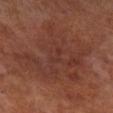The lesion was tiled from a total-body skin photograph and was not biopsied.
This is a cross-polarized tile.
A 15 mm crop from a total-body photograph taken for skin-cancer surveillance.
A male subject aged 68–72.
The lesion is on the right lower leg.
About 9.5 mm across.